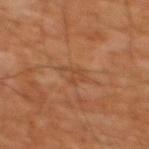  biopsy_status: not biopsied; imaged during a skin examination
  patient:
    sex: male
    age_approx: 60
  lighting: cross-polarized
  site: chest
  automated_metrics:
    eccentricity: 0.6
    shape_asymmetry: 0.65
    cielab_L: 41
    cielab_a: 21
    cielab_b: 31
    vs_skin_darker_L: 5.0
    nevus_likeness_0_100: 0
    lesion_detection_confidence_0_100: 95
  lesion_size:
    long_diameter_mm_approx: 2.5
  image:
    source: total-body photography crop
    field_of_view_mm: 15